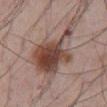<record>
<biopsy_status>not biopsied; imaged during a skin examination</biopsy_status>
<site>abdomen</site>
<automated_metrics>
  <area_mm2_approx>25.0</area_mm2_approx>
  <eccentricity>0.75</eccentricity>
  <shape_asymmetry>0.5</shape_asymmetry>
  <cielab_L>45</cielab_L>
  <cielab_a>18</cielab_a>
  <cielab_b>23</cielab_b>
  <vs_skin_contrast_norm>10.5</vs_skin_contrast_norm>
</automated_metrics>
<image>
  <source>total-body photography crop</source>
  <field_of_view_mm>15</field_of_view_mm>
</image>
<patient>
  <sex>male</sex>
  <age_approx>55</age_approx>
</patient>
</record>